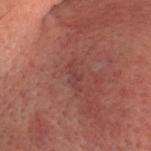  biopsy_status: not biopsied; imaged during a skin examination
  site: head or neck
  patient:
    sex: male
    age_approx: 70
  lighting: cross-polarized
  image:
    source: total-body photography crop
    field_of_view_mm: 15
  automated_metrics:
    area_mm2_approx: 4.0
    shape_asymmetry: 0.35
  lesion_size:
    long_diameter_mm_approx: 4.0